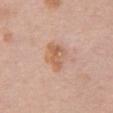{"biopsy_status": "not biopsied; imaged during a skin examination", "patient": {"sex": "female", "age_approx": 60}, "site": "front of the torso", "image": {"source": "total-body photography crop", "field_of_view_mm": 15}}